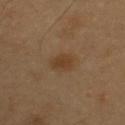Q: Was this lesion biopsied?
A: imaged on a skin check; not biopsied
Q: What lighting was used for the tile?
A: cross-polarized
Q: What is the anatomic site?
A: the chest
Q: What kind of image is this?
A: 15 mm crop, total-body photography
Q: What are the patient's age and sex?
A: male, in their mid- to late 50s